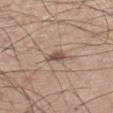Assessment:
Captured during whole-body skin photography for melanoma surveillance; the lesion was not biopsied.
Clinical summary:
Longest diameter approximately 2.5 mm. Cropped from a total-body skin-imaging series; the visible field is about 15 mm. From the left lower leg. A male patient roughly 55 years of age.